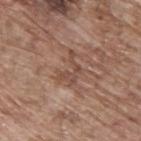notes: catalogued during a skin exam; not biopsied
illumination: white-light
automated metrics: an area of roughly 5.5 mm², a shape eccentricity near 0.75, and a shape-asymmetry score of about 0.65 (0 = symmetric); a lesion–skin lightness drop of about 8 and a normalized border contrast of about 6; border irregularity of about 9 on a 0–10 scale, a color-variation rating of about 1/10, and peripheral color asymmetry of about 0.5; lesion-presence confidence of about 60/100
acquisition: ~15 mm tile from a whole-body skin photo
body site: the upper back
subject: male, approximately 70 years of age